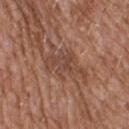• follow-up · imaged on a skin check; not biopsied